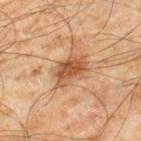Part of a total-body skin-imaging series; this lesion was reviewed on a skin check and was not flagged for biopsy. Cropped from a total-body skin-imaging series; the visible field is about 15 mm. A male subject, in their mid-40s. Located on the right lower leg.